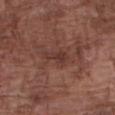Notes:
• follow-up — no biopsy performed (imaged during a skin exam)
• anatomic site — the right forearm
• lesion diameter — ~3 mm (longest diameter)
• lighting — white-light illumination
• subject — male, in their mid-70s
• image source — 15 mm crop, total-body photography